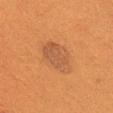Impression:
Recorded during total-body skin imaging; not selected for excision or biopsy.
Context:
The lesion's longest dimension is about 5 mm. A female subject, roughly 40 years of age. This image is a 15 mm lesion crop taken from a total-body photograph. The lesion is on the front of the torso. An algorithmic analysis of the crop reported a footprint of about 11 mm², an outline eccentricity of about 0.8 (0 = round, 1 = elongated), and a symmetry-axis asymmetry near 0.2. It also reported a border-irregularity index near 2/10 and a color-variation rating of about 2.5/10. And it measured a nevus-likeness score of about 55/100 and a detector confidence of about 100 out of 100 that the crop contains a lesion. The tile uses cross-polarized illumination.